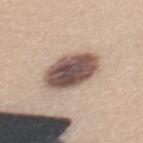Acquisition and patient details: Approximately 5.5 mm at its widest. This is a white-light tile. A roughly 15 mm field-of-view crop from a total-body skin photograph. The lesion-visualizer software estimated a footprint of about 19 mm² and an outline eccentricity of about 0.7 (0 = round, 1 = elongated). Located on the mid back. The subject is a female approximately 25 years of age.